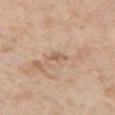Acquisition and patient details: A roughly 15 mm field-of-view crop from a total-body skin photograph. The lesion is on the right upper arm. A male patient, aged around 60.